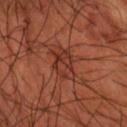image source: total-body-photography crop, ~15 mm field of view
body site: the arm
illumination: cross-polarized
subject: male, aged approximately 55